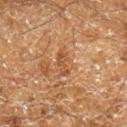Q: Was this lesion biopsied?
A: total-body-photography surveillance lesion; no biopsy
Q: What kind of image is this?
A: 15 mm crop, total-body photography
Q: What lighting was used for the tile?
A: cross-polarized illumination
Q: What is the anatomic site?
A: the right lower leg
Q: Who is the patient?
A: male, aged 58–62
Q: Automated lesion metrics?
A: an area of roughly 3.5 mm², an eccentricity of roughly 0.9, and a shape-asymmetry score of about 0.4 (0 = symmetric); an average lesion color of about L≈39 a*≈18 b*≈30 (CIELAB) and a normalized border contrast of about 6; border irregularity of about 4.5 on a 0–10 scale and peripheral color asymmetry of about 0; a classifier nevus-likeness of about 0/100